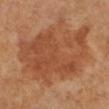Case summary:
- follow-up: catalogued during a skin exam; not biopsied
- subject: female, in their mid-50s
- image: ~15 mm crop, total-body skin-cancer survey
- site: the right lower leg